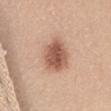{"biopsy_status": "not biopsied; imaged during a skin examination", "automated_metrics": {"cielab_L": 57, "cielab_a": 22, "cielab_b": 29, "vs_skin_darker_L": 14.0, "vs_skin_contrast_norm": 9.0, "nevus_likeness_0_100": 100, "lesion_detection_confidence_0_100": 100}, "lighting": "white-light", "site": "abdomen", "image": {"source": "total-body photography crop", "field_of_view_mm": 15}, "patient": {"sex": "female", "age_approx": 45}}